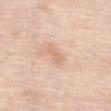| feature | finding |
|---|---|
| biopsy status | total-body-photography surveillance lesion; no biopsy |
| subject | female, aged around 70 |
| image source | ~15 mm crop, total-body skin-cancer survey |
| site | the abdomen |